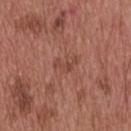This lesion was catalogued during total-body skin photography and was not selected for biopsy.
A 15 mm close-up tile from a total-body photography series done for melanoma screening.
Located on the head or neck.
This is a white-light tile.
The recorded lesion diameter is about 3 mm.
A male patient aged approximately 55.